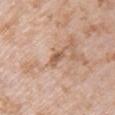A 15 mm close-up extracted from a 3D total-body photography capture. Measured at roughly 3 mm in maximum diameter. A female patient about 70 years old. The lesion is located on the arm.